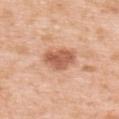This lesion was catalogued during total-body skin photography and was not selected for biopsy.
A 15 mm crop from a total-body photograph taken for skin-cancer surveillance.
The patient is a female approximately 40 years of age.
The tile uses white-light illumination.
The lesion is on the upper back.